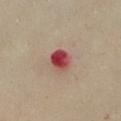Clinical impression:
Recorded during total-body skin imaging; not selected for excision or biopsy.
Context:
Measured at roughly 2.5 mm in maximum diameter. A 15 mm close-up tile from a total-body photography series done for melanoma screening. An algorithmic analysis of the crop reported a border-irregularity rating of about 1/10, internal color variation of about 9.5 on a 0–10 scale, and peripheral color asymmetry of about 3. And it measured a classifier nevus-likeness of about 0/100 and a detector confidence of about 100 out of 100 that the crop contains a lesion. On the chest. A female subject, in their mid- to late 50s.